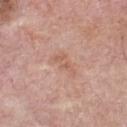Image and clinical context: This image is a 15 mm lesion crop taken from a total-body photograph. Located on the chest. Imaged with white-light lighting. The lesion's longest dimension is about 3.5 mm. The lesion-visualizer software estimated an area of roughly 5 mm² and a shape eccentricity near 0.85. The analysis additionally found an average lesion color of about L≈60 a*≈21 b*≈29 (CIELAB) and about 6 CIELAB-L* units darker than the surrounding skin. The software also gave a border-irregularity rating of about 5.5/10 and a peripheral color-asymmetry measure near 1. A male patient, aged approximately 75.